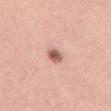This lesion was catalogued during total-body skin photography and was not selected for biopsy.
On the abdomen.
Captured under white-light illumination.
The lesion's longest dimension is about 2 mm.
This image is a 15 mm lesion crop taken from a total-body photograph.
A male patient aged 38–42.